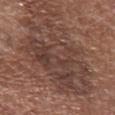Captured during whole-body skin photography for melanoma surveillance; the lesion was not biopsied.